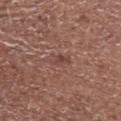  biopsy_status: not biopsied; imaged during a skin examination
  site: abdomen
  lesion_size:
    long_diameter_mm_approx: 2.5
  patient:
    sex: male
    age_approx: 70
  image:
    source: total-body photography crop
    field_of_view_mm: 15